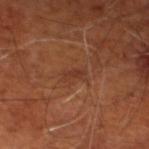Assessment: Recorded during total-body skin imaging; not selected for excision or biopsy. Image and clinical context: Cropped from a total-body skin-imaging series; the visible field is about 15 mm. A subject approximately 65 years of age. Measured at roughly 2.5 mm in maximum diameter. Imaged with cross-polarized lighting. On the left lower leg.